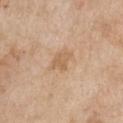The lesion was tiled from a total-body skin photograph and was not biopsied.
A roughly 15 mm field-of-view crop from a total-body skin photograph.
A female subject aged around 65.
From the chest.